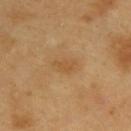Impression: Recorded during total-body skin imaging; not selected for excision or biopsy. Image and clinical context: A roughly 15 mm field-of-view crop from a total-body skin photograph. The patient is a male aged 58–62. Imaged with cross-polarized lighting. Automated image analysis of the tile measured a footprint of about 3 mm² and an outline eccentricity of about 0.8 (0 = round, 1 = elongated). The software also gave a lesion color around L≈51 a*≈19 b*≈39 in CIELAB, a lesion–skin lightness drop of about 6, and a lesion-to-skin contrast of about 5 (normalized; higher = more distinct). It also reported a classifier nevus-likeness of about 5/100. Located on the upper back. The lesion's longest dimension is about 2.5 mm.